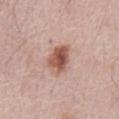notes = catalogued during a skin exam; not biopsied
tile lighting = white-light
acquisition = ~15 mm tile from a whole-body skin photo
lesion diameter = about 4 mm
location = the abdomen
subject = male, approximately 65 years of age